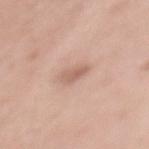Part of a total-body skin-imaging series; this lesion was reviewed on a skin check and was not flagged for biopsy.
Captured under white-light illumination.
Automated tile analysis of the lesion measured a footprint of about 3.5 mm², a shape eccentricity near 0.8, and a shape-asymmetry score of about 0.25 (0 = symmetric). The software also gave an average lesion color of about L≈61 a*≈20 b*≈27 (CIELAB) and a lesion-to-skin contrast of about 6 (normalized; higher = more distinct).
A 15 mm close-up extracted from a 3D total-body photography capture.
The subject is a female aged around 35.
Approximately 2.5 mm at its widest.
Located on the mid back.